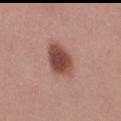The lesion was photographed on a routine skin check and not biopsied; there is no pathology result.